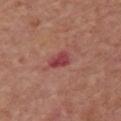Part of a total-body skin-imaging series; this lesion was reviewed on a skin check and was not flagged for biopsy.
A male patient in their mid-70s.
A 15 mm crop from a total-body photograph taken for skin-cancer surveillance.
Longest diameter approximately 3 mm.
Located on the chest.